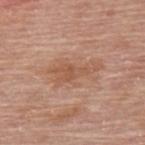Recorded during total-body skin imaging; not selected for excision or biopsy.
The subject is a male roughly 80 years of age.
On the upper back.
Cropped from a total-body skin-imaging series; the visible field is about 15 mm.